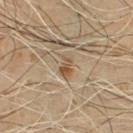Impression:
No biopsy was performed on this lesion — it was imaged during a full skin examination and was not determined to be concerning.